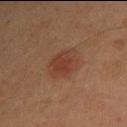biopsy status: catalogued during a skin exam; not biopsied | site: the right upper arm | size: ≈4 mm | patient: male, roughly 45 years of age | image source: ~15 mm tile from a whole-body skin photo.